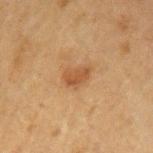The lesion was tiled from a total-body skin photograph and was not biopsied.
The lesion is located on the left upper arm.
Measured at roughly 3 mm in maximum diameter.
A close-up tile cropped from a whole-body skin photograph, about 15 mm across.
The lesion-visualizer software estimated a footprint of about 5 mm², an outline eccentricity of about 0.75 (0 = round, 1 = elongated), and two-axis asymmetry of about 0.3. It also reported a border-irregularity index near 3/10, internal color variation of about 1.5 on a 0–10 scale, and peripheral color asymmetry of about 0.5.
Captured under cross-polarized illumination.
The patient is a male roughly 50 years of age.